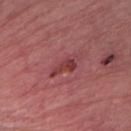Recorded during total-body skin imaging; not selected for excision or biopsy.
From the arm.
A 15 mm close-up extracted from a 3D total-body photography capture.
A male subject aged 78–82.
Captured under white-light illumination.
Longest diameter approximately 3.5 mm.